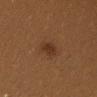<record>
<biopsy_status>not biopsied; imaged during a skin examination</biopsy_status>
</record>